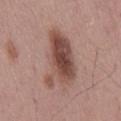Q: Was a biopsy performed?
A: imaged on a skin check; not biopsied
Q: What did automated image analysis measure?
A: a lesion area of about 21 mm², a shape eccentricity near 0.8, and a symmetry-axis asymmetry near 0.35; a detector confidence of about 100 out of 100 that the crop contains a lesion
Q: Patient demographics?
A: male, in their mid-50s
Q: Where on the body is the lesion?
A: the mid back
Q: Lesion size?
A: about 7 mm
Q: Illumination type?
A: white-light illumination
Q: How was this image acquired?
A: ~15 mm tile from a whole-body skin photo